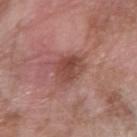Clinical impression:
No biopsy was performed on this lesion — it was imaged during a full skin examination and was not determined to be concerning.
Context:
Located on the right forearm. Approximately 4 mm at its widest. A 15 mm crop from a total-body photograph taken for skin-cancer surveillance. Imaged with white-light lighting. A female patient approximately 75 years of age.